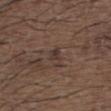workup = total-body-photography surveillance lesion; no biopsy | tile lighting = white-light illumination | size = ≈2.5 mm | patient = male, approximately 50 years of age | acquisition = total-body-photography crop, ~15 mm field of view | site = the upper back | automated lesion analysis = a border-irregularity index near 3/10, internal color variation of about 2 on a 0–10 scale, and radial color variation of about 0.5.